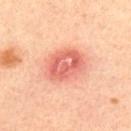| field | value |
|---|---|
| subject | male, approximately 35 years of age |
| lesion diameter | ≈5 mm |
| location | the upper back |
| illumination | cross-polarized |
| TBP lesion metrics | an area of roughly 14 mm², a shape eccentricity near 0.7, and a symmetry-axis asymmetry near 0.15; a mean CIELAB color near L≈63 a*≈32 b*≈32, about 12 CIELAB-L* units darker than the surrounding skin, and a lesion-to-skin contrast of about 7.5 (normalized; higher = more distinct); border irregularity of about 1.5 on a 0–10 scale, a within-lesion color-variation index near 5.5/10, and a peripheral color-asymmetry measure near 2; an automated nevus-likeness rating near 0 out of 100 and lesion-presence confidence of about 100/100 |
| image source | 15 mm crop, total-body photography |